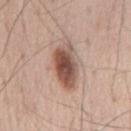Clinical impression: Imaged during a routine full-body skin examination; the lesion was not biopsied and no histopathology is available. Context: The tile uses white-light illumination. From the chest. Automated tile analysis of the lesion measured an area of roughly 13 mm², a shape eccentricity near 0.9, and a shape-asymmetry score of about 0.2 (0 = symmetric). The analysis additionally found a lesion color around L≈52 a*≈19 b*≈25 in CIELAB, roughly 16 lightness units darker than nearby skin, and a normalized lesion–skin contrast near 10.5. It also reported peripheral color asymmetry of about 2. And it measured an automated nevus-likeness rating near 100 out of 100 and lesion-presence confidence of about 100/100. The subject is a male approximately 65 years of age. A 15 mm close-up extracted from a 3D total-body photography capture. The recorded lesion diameter is about 6.5 mm.